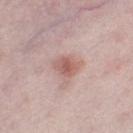Impression: Recorded during total-body skin imaging; not selected for excision or biopsy. Image and clinical context: The lesion's longest dimension is about 3 mm. The total-body-photography lesion software estimated a footprint of about 5.5 mm², an eccentricity of roughly 0.65, and a symmetry-axis asymmetry near 0.2. The analysis additionally found about 10 CIELAB-L* units darker than the surrounding skin and a normalized lesion–skin contrast near 7. The software also gave a color-variation rating of about 4/10. The lesion is on the left thigh. A female patient aged approximately 45. Cropped from a whole-body photographic skin survey; the tile spans about 15 mm. Imaged with white-light lighting.